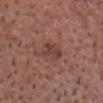Part of a total-body skin-imaging series; this lesion was reviewed on a skin check and was not flagged for biopsy. Captured under white-light illumination. This image is a 15 mm lesion crop taken from a total-body photograph. On the head or neck. Measured at roughly 3 mm in maximum diameter. The subject is a male aged approximately 65.